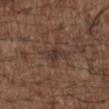follow-up: no biopsy performed (imaged during a skin exam); site: the upper back; tile lighting: white-light illumination; subject: male, about 50 years old; imaging modality: ~15 mm tile from a whole-body skin photo.